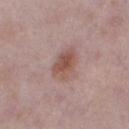follow-up: catalogued during a skin exam; not biopsied | automated lesion analysis: a lesion area of about 7.5 mm², a shape eccentricity near 0.75, and a shape-asymmetry score of about 0.25 (0 = symmetric); a mean CIELAB color near L≈52 a*≈21 b*≈24 and a normalized border contrast of about 8 | patient: female, roughly 30 years of age | image: total-body-photography crop, ~15 mm field of view | site: the right thigh | diameter: ≈4 mm | illumination: white-light.